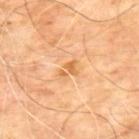Q: What is the imaging modality?
A: total-body-photography crop, ~15 mm field of view
Q: What is the anatomic site?
A: the upper back
Q: Patient demographics?
A: male, in their mid- to late 60s
Q: Lesion size?
A: ~2.5 mm (longest diameter)